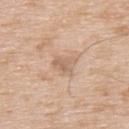Clinical summary:
Imaged with white-light lighting. On the upper back. Cropped from a whole-body photographic skin survey; the tile spans about 15 mm. The subject is a male approximately 65 years of age. About 3 mm across.